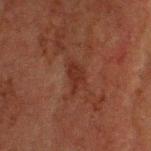The lesion was tiled from a total-body skin photograph and was not biopsied. A male subject aged approximately 60. Measured at roughly 3.5 mm in maximum diameter. A close-up tile cropped from a whole-body skin photograph, about 15 mm across. Located on the head or neck. Imaged with cross-polarized lighting.